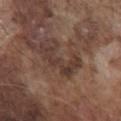Q: Was this lesion biopsied?
A: imaged on a skin check; not biopsied
Q: Lesion location?
A: the front of the torso
Q: Patient demographics?
A: male, aged around 75
Q: What kind of image is this?
A: 15 mm crop, total-body photography
Q: How was the tile lit?
A: white-light illumination
Q: Automated lesion metrics?
A: a shape-asymmetry score of about 0.6 (0 = symmetric); an average lesion color of about L≈36 a*≈17 b*≈22 (CIELAB), a lesion–skin lightness drop of about 8, and a lesion-to-skin contrast of about 7 (normalized; higher = more distinct)
Q: How large is the lesion?
A: about 5.5 mm